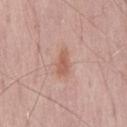Part of a total-body skin-imaging series; this lesion was reviewed on a skin check and was not flagged for biopsy. The subject is a male roughly 60 years of age. The lesion is located on the lower back. Cropped from a total-body skin-imaging series; the visible field is about 15 mm.